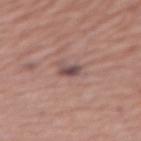Located on the leg. A female patient, about 50 years old. A region of skin cropped from a whole-body photographic capture, roughly 15 mm wide. About 2.5 mm across. Automated tile analysis of the lesion measured a lesion color around L≈46 a*≈18 b*≈18 in CIELAB and a normalized border contrast of about 10. It also reported a nevus-likeness score of about 0/100 and lesion-presence confidence of about 100/100.